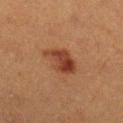biopsy status = catalogued during a skin exam; not biopsied